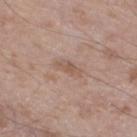Imaged during a routine full-body skin examination; the lesion was not biopsied and no histopathology is available.
A male subject aged 48 to 52.
A close-up tile cropped from a whole-body skin photograph, about 15 mm across.
Measured at roughly 3 mm in maximum diameter.
The lesion is on the left lower leg.
Captured under white-light illumination.